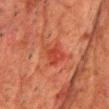Q: Is there a histopathology result?
A: total-body-photography surveillance lesion; no biopsy
Q: What is the imaging modality?
A: ~15 mm crop, total-body skin-cancer survey
Q: What is the lesion's diameter?
A: ≈3.5 mm
Q: How was the tile lit?
A: cross-polarized illumination
Q: Where on the body is the lesion?
A: the chest
Q: Automated lesion metrics?
A: a lesion area of about 6.5 mm² and a shape-asymmetry score of about 0.45 (0 = symmetric); a lesion color around L≈36 a*≈30 b*≈30 in CIELAB, a lesion–skin lightness drop of about 6, and a normalized border contrast of about 6; a nevus-likeness score of about 25/100 and a lesion-detection confidence of about 100/100
Q: Who is the patient?
A: male, in their 60s